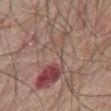- follow-up · catalogued during a skin exam; not biopsied
- location · the chest
- lesion diameter · about 11.5 mm
- subject · male, aged 78–82
- acquisition · ~15 mm crop, total-body skin-cancer survey
- lighting · white-light